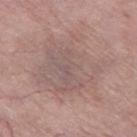biopsy_status: not biopsied; imaged during a skin examination
lighting: white-light
patient:
  sex: female
  age_approx: 75
lesion_size:
  long_diameter_mm_approx: 9.0
image:
  source: total-body photography crop
  field_of_view_mm: 15
site: left thigh